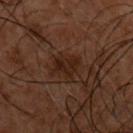  patient:
    sex: male
    age_approx: 50
  image:
    source: total-body photography crop
    field_of_view_mm: 15
  lesion_size:
    long_diameter_mm_approx: 4.0
  automated_metrics:
    area_mm2_approx: 6.5
    eccentricity: 0.8
    shape_asymmetry: 0.3
    vs_skin_darker_L: 5.0
    border_irregularity_0_10: 3.0
    color_variation_0_10: 2.5
    peripheral_color_asymmetry: 1.0
    nevus_likeness_0_100: 5
    lesion_detection_confidence_0_100: 90
  site: chest
  lighting: cross-polarized